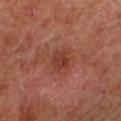workup: catalogued during a skin exam; not biopsied | tile lighting: cross-polarized illumination | diameter: ≈3 mm | patient: male, aged 63–67 | location: the left lower leg | imaging modality: ~15 mm tile from a whole-body skin photo | automated metrics: an area of roughly 5 mm² and an outline eccentricity of about 0.65 (0 = round, 1 = elongated); a classifier nevus-likeness of about 0/100 and a lesion-detection confidence of about 100/100.